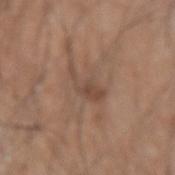  biopsy_status: not biopsied; imaged during a skin examination
  automated_metrics:
    area_mm2_approx: 5.0
    eccentricity: 0.9
    cielab_L: 46
    cielab_a: 17
    cielab_b: 27
    vs_skin_darker_L: 8.0
  site: left upper arm
  patient:
    sex: male
    age_approx: 60
  lighting: white-light
  image:
    source: total-body photography crop
    field_of_view_mm: 15
  lesion_size:
    long_diameter_mm_approx: 4.0